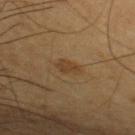This lesion was catalogued during total-body skin photography and was not selected for biopsy.
A male patient, roughly 60 years of age.
Automated tile analysis of the lesion measured a mean CIELAB color near L≈35 a*≈15 b*≈30.
The tile uses cross-polarized illumination.
A lesion tile, about 15 mm wide, cut from a 3D total-body photograph.
The lesion is located on the left upper arm.